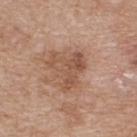Recorded during total-body skin imaging; not selected for excision or biopsy.
A female patient about 40 years old.
The tile uses white-light illumination.
Measured at roughly 5 mm in maximum diameter.
The lesion is located on the upper back.
A roughly 15 mm field-of-view crop from a total-body skin photograph.